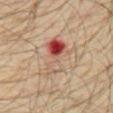Part of a total-body skin-imaging series; this lesion was reviewed on a skin check and was not flagged for biopsy. Imaged with cross-polarized lighting. Measured at roughly 6 mm in maximum diameter. A close-up tile cropped from a whole-body skin photograph, about 15 mm across. The lesion is located on the front of the torso. A male patient aged 28–32.